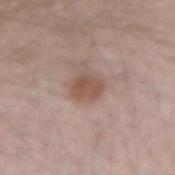{
  "biopsy_status": "not biopsied; imaged during a skin examination",
  "site": "right forearm",
  "image": {
    "source": "total-body photography crop",
    "field_of_view_mm": 15
  },
  "patient": {
    "sex": "male",
    "age_approx": 40
  },
  "lighting": "white-light"
}